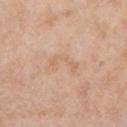biopsy status: imaged on a skin check; not biopsied
lesion diameter: ~4 mm (longest diameter)
site: the right upper arm
tile lighting: white-light
patient: female, in their 70s
imaging modality: total-body-photography crop, ~15 mm field of view